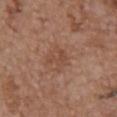Image and clinical context:
The lesion is on the chest. A close-up tile cropped from a whole-body skin photograph, about 15 mm across. Measured at roughly 2.5 mm in maximum diameter. The subject is a female aged around 65.